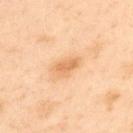Imaged during a routine full-body skin examination; the lesion was not biopsied and no histopathology is available. The lesion is on the upper back. A 15 mm crop from a total-body photograph taken for skin-cancer surveillance. The subject is a male roughly 55 years of age.